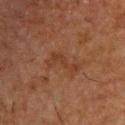notes: total-body-photography surveillance lesion; no biopsy | body site: the upper back | illumination: cross-polarized | acquisition: total-body-photography crop, ~15 mm field of view | patient: male, aged approximately 50 | lesion size: ≈5 mm.